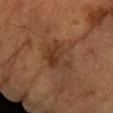Captured during whole-body skin photography for melanoma surveillance; the lesion was not biopsied. A female subject about 60 years old. A close-up tile cropped from a whole-body skin photograph, about 15 mm across. Approximately 3.5 mm at its widest. On the left forearm.